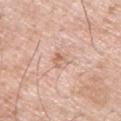Impression: No biopsy was performed on this lesion — it was imaged during a full skin examination and was not determined to be concerning. Context: Imaged with white-light lighting. The patient is a male approximately 65 years of age. About 3 mm across. A region of skin cropped from a whole-body photographic capture, roughly 15 mm wide. Located on the right upper arm.